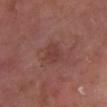{"biopsy_status": "not biopsied; imaged during a skin examination", "lighting": "white-light", "automated_metrics": {"cielab_L": 40, "cielab_a": 24, "cielab_b": 24, "vs_skin_darker_L": 6.0, "vs_skin_contrast_norm": 5.0, "border_irregularity_0_10": 2.0, "color_variation_0_10": 1.5}, "site": "right lower leg", "patient": {"sex": "male", "age_approx": 65}, "image": {"source": "total-body photography crop", "field_of_view_mm": 15}}